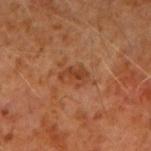Captured during whole-body skin photography for melanoma surveillance; the lesion was not biopsied. A 15 mm crop from a total-body photograph taken for skin-cancer surveillance. From the arm. The tile uses cross-polarized illumination. A male patient, aged 58 to 62. The lesion's longest dimension is about 3 mm.